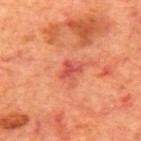{"biopsy_status": "not biopsied; imaged during a skin examination", "image": {"source": "total-body photography crop", "field_of_view_mm": 15}, "lesion_size": {"long_diameter_mm_approx": 2.5}, "patient": {"sex": "male", "age_approx": 70}, "site": "back", "lighting": "cross-polarized"}